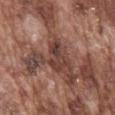Impression: This lesion was catalogued during total-body skin photography and was not selected for biopsy. Acquisition and patient details: The lesion is located on the mid back. Captured under white-light illumination. About 3.5 mm across. The total-body-photography lesion software estimated an average lesion color of about L≈39 a*≈21 b*≈23 (CIELAB), roughly 10 lightness units darker than nearby skin, and a normalized border contrast of about 8.5. And it measured an automated nevus-likeness rating near 0 out of 100. A 15 mm close-up extracted from a 3D total-body photography capture. A male patient aged around 75.